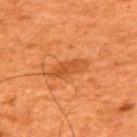notes: imaged on a skin check; not biopsied
body site: the upper back
lesion diameter: about 4.5 mm
tile lighting: cross-polarized
TBP lesion metrics: about 7 CIELAB-L* units darker than the surrounding skin
image source: total-body-photography crop, ~15 mm field of view
patient: male, about 60 years old